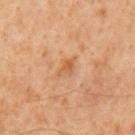Imaged during a routine full-body skin examination; the lesion was not biopsied and no histopathology is available.
Captured under cross-polarized illumination.
Cropped from a total-body skin-imaging series; the visible field is about 15 mm.
From the mid back.
A male patient aged around 60.